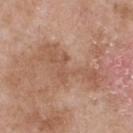Impression:
Part of a total-body skin-imaging series; this lesion was reviewed on a skin check and was not flagged for biopsy.
Context:
On the upper back. Approximately 8 mm at its widest. A male patient aged approximately 55. A roughly 15 mm field-of-view crop from a total-body skin photograph. Imaged with white-light lighting.